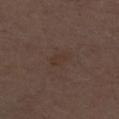Impression:
Recorded during total-body skin imaging; not selected for excision or biopsy.
Context:
This is a white-light tile. Cropped from a whole-body photographic skin survey; the tile spans about 15 mm. A male patient, aged around 70. Approximately 3 mm at its widest. On the right thigh.